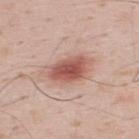workup = imaged on a skin check; not biopsied
imaging modality = 15 mm crop, total-body photography
site = the upper back
lesion size = ~4.5 mm (longest diameter)
lighting = white-light illumination
patient = male, aged 33 to 37
automated lesion analysis = a classifier nevus-likeness of about 100/100 and a lesion-detection confidence of about 100/100